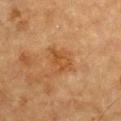Recorded during total-body skin imaging; not selected for excision or biopsy. Located on the chest. The total-body-photography lesion software estimated border irregularity of about 4.5 on a 0–10 scale and a peripheral color-asymmetry measure near 1. The subject is a male aged 83 or over. Cropped from a total-body skin-imaging series; the visible field is about 15 mm. The tile uses cross-polarized illumination.